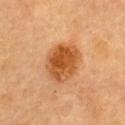follow-up: no biopsy performed (imaged during a skin exam)
imaging modality: ~15 mm crop, total-body skin-cancer survey
size: ~5 mm (longest diameter)
body site: the upper back
lighting: cross-polarized illumination
patient: female, about 60 years old